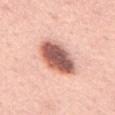Acquisition and patient details:
The lesion is on the abdomen. The lesion-visualizer software estimated an area of roughly 16 mm², an outline eccentricity of about 0.8 (0 = round, 1 = elongated), and two-axis asymmetry of about 0.15. And it measured an average lesion color of about L≈59 a*≈25 b*≈27 (CIELAB), a lesion–skin lightness drop of about 21, and a normalized border contrast of about 12.5. Imaged with white-light lighting. Approximately 5.5 mm at its widest. A 15 mm close-up extracted from a 3D total-body photography capture. A male patient approximately 60 years of age.